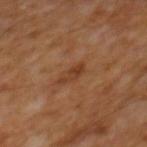Case summary:
* subject — male, about 65 years old
* imaging modality — total-body-photography crop, ~15 mm field of view
* body site — the mid back